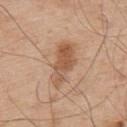Imaged during a routine full-body skin examination; the lesion was not biopsied and no histopathology is available. Cropped from a whole-body photographic skin survey; the tile spans about 15 mm. A male patient approximately 55 years of age. Automated tile analysis of the lesion measured a lesion area of about 12 mm², a shape eccentricity near 0.9, and two-axis asymmetry of about 0.25. It also reported roughly 10 lightness units darker than nearby skin and a normalized border contrast of about 7. This is a white-light tile. Located on the upper back. Measured at roughly 5.5 mm in maximum diameter.